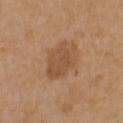<lesion>
  <biopsy_status>not biopsied; imaged during a skin examination</biopsy_status>
  <lighting>white-light</lighting>
  <site>left upper arm</site>
  <patient>
    <sex>female</sex>
    <age_approx>40</age_approx>
  </patient>
  <automated_metrics>
    <area_mm2_approx>12.0</area_mm2_approx>
    <eccentricity>0.65</eccentricity>
    <color_variation_0_10>2.5</color_variation_0_10>
    <peripheral_color_asymmetry>1.0</peripheral_color_asymmetry>
  </automated_metrics>
  <image>
    <source>total-body photography crop</source>
    <field_of_view_mm>15</field_of_view_mm>
  </image>
</lesion>